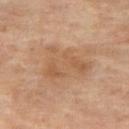Q: Is there a histopathology result?
A: total-body-photography surveillance lesion; no biopsy
Q: Patient demographics?
A: female, aged 63–67
Q: How was this image acquired?
A: ~15 mm crop, total-body skin-cancer survey
Q: Lesion location?
A: the right thigh